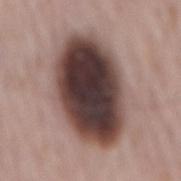Automated tile analysis of the lesion measured about 24 CIELAB-L* units darker than the surrounding skin and a lesion-to-skin contrast of about 17 (normalized; higher = more distinct). The software also gave a border-irregularity rating of about 1.5/10, internal color variation of about 9 on a 0–10 scale, and a peripheral color-asymmetry measure near 2.5. The lesion is on the mid back. Imaged with white-light lighting. A 15 mm crop from a total-body photograph taken for skin-cancer surveillance. About 9.5 mm across. A male patient, in their mid-60s.